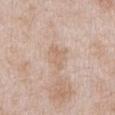biopsy status: catalogued during a skin exam; not biopsied
acquisition: total-body-photography crop, ~15 mm field of view
size: about 3.5 mm
automated metrics: a footprint of about 4 mm², a shape eccentricity near 0.9, and a symmetry-axis asymmetry near 0.45; border irregularity of about 5.5 on a 0–10 scale, a color-variation rating of about 1/10, and peripheral color asymmetry of about 0.5; an automated nevus-likeness rating near 0 out of 100
tile lighting: white-light
site: the front of the torso
subject: male, about 50 years old